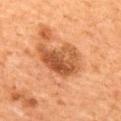workup: no biopsy performed (imaged during a skin exam).